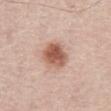Q: Was a biopsy performed?
A: catalogued during a skin exam; not biopsied
Q: Lesion location?
A: the abdomen
Q: What did automated image analysis measure?
A: a lesion–skin lightness drop of about 14 and a lesion-to-skin contrast of about 9.5 (normalized; higher = more distinct); an automated nevus-likeness rating near 100 out of 100 and a lesion-detection confidence of about 100/100
Q: What lighting was used for the tile?
A: white-light
Q: How was this image acquired?
A: ~15 mm tile from a whole-body skin photo
Q: Who is the patient?
A: male, aged 68–72